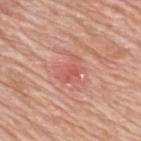Findings:
- notes — total-body-photography surveillance lesion; no biopsy
- patient — male, aged approximately 80
- imaging modality — ~15 mm tile from a whole-body skin photo
- location — the upper back